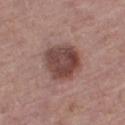Assessment: Imaged during a routine full-body skin examination; the lesion was not biopsied and no histopathology is available.